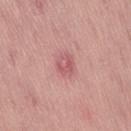Clinical impression: Part of a total-body skin-imaging series; this lesion was reviewed on a skin check and was not flagged for biopsy. Context: The patient is a male aged 53–57. Measured at roughly 3 mm in maximum diameter. The lesion is on the leg. Cropped from a total-body skin-imaging series; the visible field is about 15 mm.